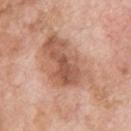The lesion was photographed on a routine skin check and not biopsied; there is no pathology result.
On the upper back.
Measured at roughly 10.5 mm in maximum diameter.
Cropped from a whole-body photographic skin survey; the tile spans about 15 mm.
Imaged with white-light lighting.
A male patient, roughly 60 years of age.